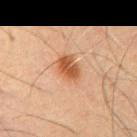This lesion was catalogued during total-body skin photography and was not selected for biopsy.
The lesion's longest dimension is about 3.5 mm.
A close-up tile cropped from a whole-body skin photograph, about 15 mm across.
A male patient, aged 53–57.
The lesion is located on the chest.